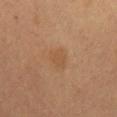| feature | finding |
|---|---|
| biopsy status | imaged on a skin check; not biopsied |
| illumination | cross-polarized illumination |
| lesion diameter | ≈3 mm |
| subject | female, roughly 60 years of age |
| image | ~15 mm crop, total-body skin-cancer survey |
| location | the mid back |